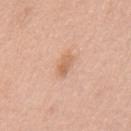follow-up: no biopsy performed (imaged during a skin exam)
illumination: white-light
subject: female, in their 60s
image source: ~15 mm tile from a whole-body skin photo
anatomic site: the mid back